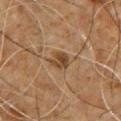biopsy status: no biopsy performed (imaged during a skin exam); subject: male, aged approximately 60; imaging modality: ~15 mm crop, total-body skin-cancer survey; illumination: cross-polarized; location: the chest.